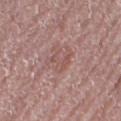Q: Was a biopsy performed?
A: catalogued during a skin exam; not biopsied
Q: How was the tile lit?
A: white-light illumination
Q: Who is the patient?
A: female, aged 63–67
Q: What is the anatomic site?
A: the right thigh
Q: How was this image acquired?
A: ~15 mm tile from a whole-body skin photo
Q: Automated lesion metrics?
A: a mean CIELAB color near L≈53 a*≈21 b*≈22, roughly 6 lightness units darker than nearby skin, and a lesion-to-skin contrast of about 5 (normalized; higher = more distinct); a border-irregularity index near 6.5/10 and peripheral color asymmetry of about 0.5